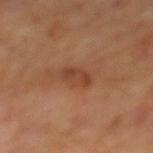Recorded during total-body skin imaging; not selected for excision or biopsy. Located on the mid back. A male subject aged 68 to 72. The lesion's longest dimension is about 3 mm. A close-up tile cropped from a whole-body skin photograph, about 15 mm across. The tile uses cross-polarized illumination.